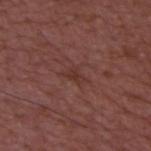Findings:
• workup — imaged on a skin check; not biopsied